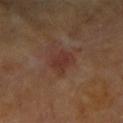This lesion was catalogued during total-body skin photography and was not selected for biopsy.
On the arm.
The lesion-visualizer software estimated a footprint of about 6 mm², a shape eccentricity near 0.7, and two-axis asymmetry of about 0.25.
The patient is a female in their mid-70s.
Cropped from a total-body skin-imaging series; the visible field is about 15 mm.
Approximately 3 mm at its widest.
The tile uses cross-polarized illumination.